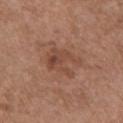Notes:
– image source: ~15 mm tile from a whole-body skin photo
– automated metrics: a footprint of about 8.5 mm² and two-axis asymmetry of about 0.5; roughly 7 lightness units darker than nearby skin; a border-irregularity index near 8/10 and a within-lesion color-variation index near 5/10
– size: about 4 mm
– lighting: white-light illumination
– patient: female, in their mid- to late 70s
– location: the chest